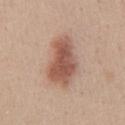lesion_size:
  long_diameter_mm_approx: 6.0
patient:
  sex: male
  age_approx: 30
image:
  source: total-body photography crop
  field_of_view_mm: 15
automated_metrics:
  area_mm2_approx: 17.0
  eccentricity: 0.85
  shape_asymmetry: 0.25
  border_irregularity_0_10: 2.5
  color_variation_0_10: 4.5
  peripheral_color_asymmetry: 1.5
lighting: white-light
site: chest